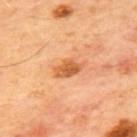{
  "biopsy_status": "not biopsied; imaged during a skin examination",
  "lesion_size": {
    "long_diameter_mm_approx": 4.0
  },
  "automated_metrics": {
    "area_mm2_approx": 6.0,
    "eccentricity": 0.85,
    "shape_asymmetry": 0.25,
    "border_irregularity_0_10": 2.5,
    "color_variation_0_10": 3.0,
    "peripheral_color_asymmetry": 1.0
  },
  "image": {
    "source": "total-body photography crop",
    "field_of_view_mm": 15
  },
  "lighting": "cross-polarized",
  "site": "mid back",
  "patient": {
    "sex": "male",
    "age_approx": 65
  }
}